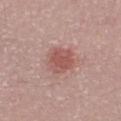Assessment:
The lesion was photographed on a routine skin check and not biopsied; there is no pathology result.
Image and clinical context:
This is a white-light tile. Automated tile analysis of the lesion measured a shape eccentricity near 0.65 and a shape-asymmetry score of about 0.25 (0 = symmetric). It also reported a lesion color around L≈54 a*≈24 b*≈24 in CIELAB and roughly 10 lightness units darker than nearby skin. The analysis additionally found a border-irregularity index near 2.5/10, a color-variation rating of about 2.5/10, and a peripheral color-asymmetry measure near 0.5. And it measured a nevus-likeness score of about 85/100 and lesion-presence confidence of about 100/100. On the right forearm. The lesion's longest dimension is about 4 mm. Cropped from a whole-body photographic skin survey; the tile spans about 15 mm. A male patient, aged approximately 50.